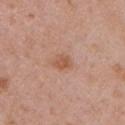Imaged during a routine full-body skin examination; the lesion was not biopsied and no histopathology is available.
Imaged with white-light lighting.
The lesion is on the left upper arm.
An algorithmic analysis of the crop reported a shape eccentricity near 0.65 and a shape-asymmetry score of about 0.3 (0 = symmetric). It also reported an automated nevus-likeness rating near 55 out of 100 and a lesion-detection confidence of about 100/100.
This image is a 15 mm lesion crop taken from a total-body photograph.
A female patient, aged 48 to 52.